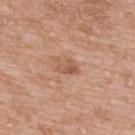imaging modality=~15 mm crop, total-body skin-cancer survey | illumination=white-light | anatomic site=the upper back | automated metrics=border irregularity of about 3.5 on a 0–10 scale, a within-lesion color-variation index near 1.5/10, and a peripheral color-asymmetry measure near 0.5; an automated nevus-likeness rating near 0 out of 100 and a detector confidence of about 100 out of 100 that the crop contains a lesion | patient=male, in their 60s | lesion size=~2.5 mm (longest diameter).